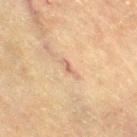Case summary:
– biopsy status — imaged on a skin check; not biopsied
– subject — female, in their 80s
– image source — ~15 mm crop, total-body skin-cancer survey
– lighting — cross-polarized illumination
– size — about 2.5 mm
– body site — the right thigh